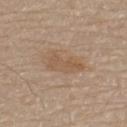The recorded lesion diameter is about 4.5 mm.
The tile uses white-light illumination.
On the upper back.
Automated image analysis of the tile measured a mean CIELAB color near L≈55 a*≈16 b*≈31, about 7 CIELAB-L* units darker than the surrounding skin, and a normalized lesion–skin contrast near 5.5. It also reported a classifier nevus-likeness of about 0/100 and a detector confidence of about 100 out of 100 that the crop contains a lesion.
Cropped from a whole-body photographic skin survey; the tile spans about 15 mm.
A male subject aged around 80.